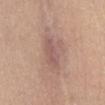Assessment: No biopsy was performed on this lesion — it was imaged during a full skin examination and was not determined to be concerning. Clinical summary: The lesion-visualizer software estimated a border-irregularity index near 4/10 and internal color variation of about 3 on a 0–10 scale. The software also gave lesion-presence confidence of about 80/100. Imaged with white-light lighting. The recorded lesion diameter is about 7 mm. This image is a 15 mm lesion crop taken from a total-body photograph. The lesion is on the front of the torso. A female subject about 45 years old.